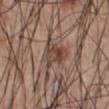This lesion was catalogued during total-body skin photography and was not selected for biopsy. The lesion-visualizer software estimated a mean CIELAB color near L≈44 a*≈17 b*≈24, a lesion–skin lightness drop of about 10, and a lesion-to-skin contrast of about 7.5 (normalized; higher = more distinct). And it measured a border-irregularity rating of about 5/10 and peripheral color asymmetry of about 2.5. It also reported an automated nevus-likeness rating near 50 out of 100 and lesion-presence confidence of about 80/100. Imaged with white-light lighting. A male subject, in their mid- to late 50s. Longest diameter approximately 4 mm. The lesion is on the abdomen. A region of skin cropped from a whole-body photographic capture, roughly 15 mm wide.